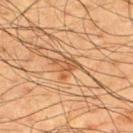Background:
A lesion tile, about 15 mm wide, cut from a 3D total-body photograph. On the upper back. A male patient, roughly 60 years of age. About 3 mm across. This is a cross-polarized tile. Automated image analysis of the tile measured a footprint of about 4.5 mm², an outline eccentricity of about 0.5 (0 = round, 1 = elongated), and a shape-asymmetry score of about 0.55 (0 = symmetric). The software also gave a lesion color around L≈43 a*≈19 b*≈32 in CIELAB and a normalized lesion–skin contrast near 6.5. The software also gave a nevus-likeness score of about 5/100 and a lesion-detection confidence of about 100/100.